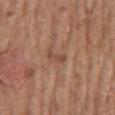Notes:
• image-analysis metrics — an eccentricity of roughly 0.9 and a symmetry-axis asymmetry near 0.4; a lesion color around L≈48 a*≈21 b*≈29 in CIELAB and a lesion–skin lightness drop of about 8; a border-irregularity rating of about 4/10 and peripheral color asymmetry of about 0
• location — the mid back
• patient — male, approximately 75 years of age
• image — ~15 mm crop, total-body skin-cancer survey